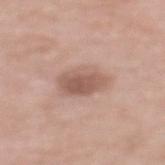No biopsy was performed on this lesion — it was imaged during a full skin examination and was not determined to be concerning. The lesion's longest dimension is about 4.5 mm. A female subject in their 70s. Captured under white-light illumination. The lesion-visualizer software estimated an eccentricity of roughly 0.75 and a shape-asymmetry score of about 0.2 (0 = symmetric). The analysis additionally found a border-irregularity rating of about 2/10 and a peripheral color-asymmetry measure near 1.5. The lesion is located on the mid back. A roughly 15 mm field-of-view crop from a total-body skin photograph.